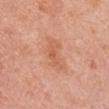Assessment:
Imaged during a routine full-body skin examination; the lesion was not biopsied and no histopathology is available.
Background:
Cropped from a total-body skin-imaging series; the visible field is about 15 mm. From the left upper arm. A female subject aged approximately 50. Automated tile analysis of the lesion measured a footprint of about 10 mm², a shape eccentricity near 0.85, and a symmetry-axis asymmetry near 0.3. The analysis additionally found a classifier nevus-likeness of about 0/100 and a lesion-detection confidence of about 100/100. Measured at roughly 5 mm in maximum diameter. This is a white-light tile.